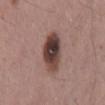Q: Was this lesion biopsied?
A: total-body-photography surveillance lesion; no biopsy
Q: What lighting was used for the tile?
A: white-light illumination
Q: Patient demographics?
A: male, aged around 55
Q: Lesion location?
A: the mid back
Q: How was this image acquired?
A: ~15 mm crop, total-body skin-cancer survey
Q: What is the lesion's diameter?
A: about 6 mm